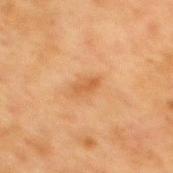workup=catalogued during a skin exam; not biopsied | image source=~15 mm crop, total-body skin-cancer survey | patient=female, about 40 years old | body site=the mid back | illumination=cross-polarized illumination | lesion size=~2.5 mm (longest diameter).